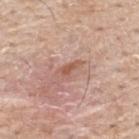Part of a total-body skin-imaging series; this lesion was reviewed on a skin check and was not flagged for biopsy. A lesion tile, about 15 mm wide, cut from a 3D total-body photograph. A male patient, about 55 years old. From the upper back. Approximately 3 mm at its widest.